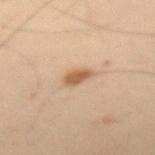Impression:
This lesion was catalogued during total-body skin photography and was not selected for biopsy.